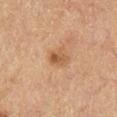The lesion was tiled from a total-body skin photograph and was not biopsied.
The patient is a male approximately 85 years of age or older.
From the right lower leg.
A roughly 15 mm field-of-view crop from a total-body skin photograph.